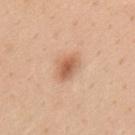| feature | finding |
|---|---|
| notes | total-body-photography surveillance lesion; no biopsy |
| image-analysis metrics | a lesion color around L≈61 a*≈23 b*≈34 in CIELAB, roughly 12 lightness units darker than nearby skin, and a normalized border contrast of about 7.5; border irregularity of about 2.5 on a 0–10 scale and internal color variation of about 3.5 on a 0–10 scale; a nevus-likeness score of about 90/100 and lesion-presence confidence of about 100/100 |
| image | ~15 mm crop, total-body skin-cancer survey |
| subject | male, aged approximately 35 |
| lighting | white-light |
| site | the mid back |